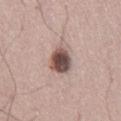| feature | finding |
|---|---|
| follow-up | no biopsy performed (imaged during a skin exam) |
| imaging modality | ~15 mm crop, total-body skin-cancer survey |
| tile lighting | white-light |
| patient | male, about 45 years old |
| location | the leg |
| lesion diameter | ≈3.5 mm |